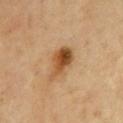The lesion was photographed on a routine skin check and not biopsied; there is no pathology result.
Automated tile analysis of the lesion measured a nevus-likeness score of about 95/100 and a detector confidence of about 100 out of 100 that the crop contains a lesion.
The lesion's longest dimension is about 4.5 mm.
On the front of the torso.
A lesion tile, about 15 mm wide, cut from a 3D total-body photograph.
This is a cross-polarized tile.
The patient is a male roughly 70 years of age.